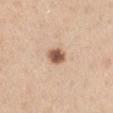Assessment: The lesion was photographed on a routine skin check and not biopsied; there is no pathology result. Image and clinical context: From the left upper arm. This is a white-light tile. A lesion tile, about 15 mm wide, cut from a 3D total-body photograph. A male subject, in their 60s. Longest diameter approximately 2.5 mm.